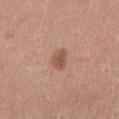Impression:
The lesion was photographed on a routine skin check and not biopsied; there is no pathology result.
Clinical summary:
Captured under white-light illumination. The subject is a male in their 40s. Automated image analysis of the tile measured a lesion area of about 4 mm², an eccentricity of roughly 0.65, and two-axis asymmetry of about 0.3. It also reported a lesion-detection confidence of about 100/100. A close-up tile cropped from a whole-body skin photograph, about 15 mm across. Located on the mid back. About 2.5 mm across.